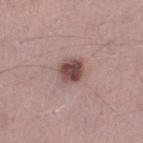The lesion was tiled from a total-body skin photograph and was not biopsied. A male patient in their 50s. A roughly 15 mm field-of-view crop from a total-body skin photograph. The lesion is located on the left lower leg.